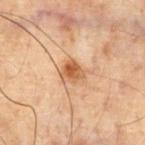Case summary:
• biopsy status: imaged on a skin check; not biopsied
• imaging modality: ~15 mm crop, total-body skin-cancer survey
• patient: male, approximately 70 years of age
• site: the chest
• lighting: cross-polarized